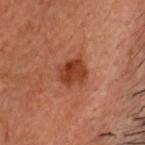workup=total-body-photography surveillance lesion; no biopsy
location=the head or neck
image=15 mm crop, total-body photography
subject=male, about 65 years old